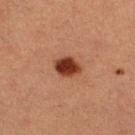  biopsy_status: not biopsied; imaged during a skin examination
  site: right lower leg
  patient:
    sex: female
    age_approx: 40
  image:
    source: total-body photography crop
    field_of_view_mm: 15
  lesion_size:
    long_diameter_mm_approx: 3.5
  automated_metrics:
    area_mm2_approx: 6.5
    eccentricity: 0.7
    shape_asymmetry: 0.15
    nevus_likeness_0_100: 100